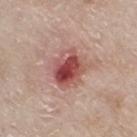notes: imaged on a skin check; not biopsied
automated metrics: peripheral color asymmetry of about 3.5; a classifier nevus-likeness of about 5/100
image: total-body-photography crop, ~15 mm field of view
body site: the right thigh
lesion size: ~4 mm (longest diameter)
lighting: white-light illumination
patient: female, aged 73–77